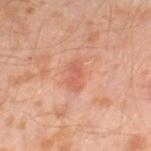notes = total-body-photography surveillance lesion; no biopsy
location = the leg
illumination = cross-polarized
imaging modality = ~15 mm tile from a whole-body skin photo
subject = male, approximately 30 years of age
size = ≈3 mm